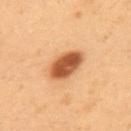{"biopsy_status": "not biopsied; imaged during a skin examination", "image": {"source": "total-body photography crop", "field_of_view_mm": 15}, "automated_metrics": {"border_irregularity_0_10": 1.5, "color_variation_0_10": 5.5, "peripheral_color_asymmetry": 1.5}, "patient": {"sex": "male", "age_approx": 55}, "site": "back"}